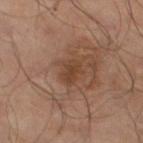Clinical impression: Part of a total-body skin-imaging series; this lesion was reviewed on a skin check and was not flagged for biopsy. Image and clinical context: Imaged with cross-polarized lighting. A close-up tile cropped from a whole-body skin photograph, about 15 mm across. On the left lower leg. The lesion's longest dimension is about 3 mm. A male subject aged 63 to 67.